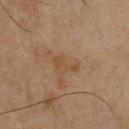Captured during whole-body skin photography for melanoma surveillance; the lesion was not biopsied. Captured under cross-polarized illumination. A lesion tile, about 15 mm wide, cut from a 3D total-body photograph. On the chest. Measured at roughly 3.5 mm in maximum diameter. A male patient, about 45 years old.